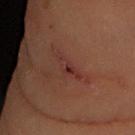  biopsy_status: not biopsied; imaged during a skin examination
  lesion_size:
    long_diameter_mm_approx: 4.0
  lighting: cross-polarized
  patient:
    sex: male
    age_approx: 55
  site: right forearm
  image:
    source: total-body photography crop
    field_of_view_mm: 15